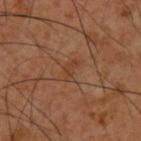Captured during whole-body skin photography for melanoma surveillance; the lesion was not biopsied.
A male subject aged 58–62.
On the upper back.
A 15 mm close-up extracted from a 3D total-body photography capture.